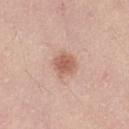image: total-body-photography crop, ~15 mm field of view
lesion size: about 3 mm
anatomic site: the left thigh
patient: female, aged around 40
image-analysis metrics: an area of roughly 6.5 mm², an eccentricity of roughly 0.3, and a symmetry-axis asymmetry near 0.2; an average lesion color of about L≈60 a*≈22 b*≈29 (CIELAB), roughly 11 lightness units darker than nearby skin, and a lesion-to-skin contrast of about 7.5 (normalized; higher = more distinct); internal color variation of about 2.5 on a 0–10 scale and peripheral color asymmetry of about 0.5
lighting: white-light